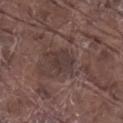| key | value |
|---|---|
| follow-up | catalogued during a skin exam; not biopsied |
| image | ~15 mm tile from a whole-body skin photo |
| body site | the right lower leg |
| size | ~3 mm (longest diameter) |
| patient | male, about 80 years old |